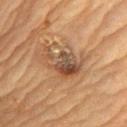The lesion was photographed on a routine skin check and not biopsied; there is no pathology result. Measured at roughly 5 mm in maximum diameter. The lesion is on the chest. Cropped from a whole-body photographic skin survey; the tile spans about 15 mm. The lesion-visualizer software estimated a border-irregularity index near 5/10 and a color-variation rating of about 9.5/10. A female patient, in their 60s.